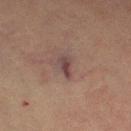Recorded during total-body skin imaging; not selected for excision or biopsy.